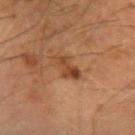This lesion was catalogued during total-body skin photography and was not selected for biopsy.
The lesion is located on the left forearm.
A close-up tile cropped from a whole-body skin photograph, about 15 mm across.
The lesion's longest dimension is about 3.5 mm.
The patient is a male aged around 60.
Captured under cross-polarized illumination.